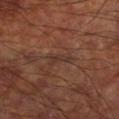| field | value |
|---|---|
| follow-up | imaged on a skin check; not biopsied |
| patient | male, aged 58 to 62 |
| diameter | ≈3 mm |
| acquisition | total-body-photography crop, ~15 mm field of view |
| location | the right lower leg |
| lighting | cross-polarized illumination |
| image-analysis metrics | a mean CIELAB color near L≈32 a*≈18 b*≈23, a lesion–skin lightness drop of about 5, and a lesion-to-skin contrast of about 6 (normalized; higher = more distinct); a classifier nevus-likeness of about 0/100 and a detector confidence of about 95 out of 100 that the crop contains a lesion |